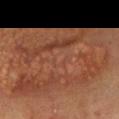<record>
<biopsy_status>not biopsied; imaged during a skin examination</biopsy_status>
<site>chest</site>
<patient>
  <sex>female</sex>
  <age_approx>40</age_approx>
</patient>
<image>
  <source>total-body photography crop</source>
  <field_of_view_mm>15</field_of_view_mm>
</image>
</record>